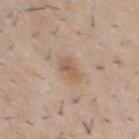{
  "biopsy_status": "not biopsied; imaged during a skin examination",
  "site": "chest",
  "patient": {
    "sex": "male",
    "age_approx": 30
  },
  "automated_metrics": {
    "area_mm2_approx": 4.5,
    "eccentricity": 0.75,
    "cielab_L": 58,
    "cielab_a": 16,
    "cielab_b": 30,
    "vs_skin_contrast_norm": 6.0,
    "border_irregularity_0_10": 3.0
  },
  "lesion_size": {
    "long_diameter_mm_approx": 3.0
  },
  "lighting": "white-light",
  "image": {
    "source": "total-body photography crop",
    "field_of_view_mm": 15
  }
}